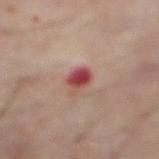biopsy status: catalogued during a skin exam; not biopsied
size: ≈3 mm
patient: male, aged 58 to 62
anatomic site: the abdomen
illumination: cross-polarized illumination
acquisition: total-body-photography crop, ~15 mm field of view